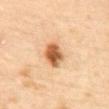No biopsy was performed on this lesion — it was imaged during a full skin examination and was not determined to be concerning. A female patient, aged approximately 60. This image is a 15 mm lesion crop taken from a total-body photograph. Imaged with cross-polarized lighting. The recorded lesion diameter is about 4 mm. The lesion is located on the mid back.